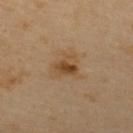Clinical impression:
Part of a total-body skin-imaging series; this lesion was reviewed on a skin check and was not flagged for biopsy.
Image and clinical context:
The recorded lesion diameter is about 3 mm. The subject is a male about 55 years old. This image is a 15 mm lesion crop taken from a total-body photograph. Located on the upper back. The lesion-visualizer software estimated a mean CIELAB color near L≈47 a*≈19 b*≈36, about 10 CIELAB-L* units darker than the surrounding skin, and a normalized border contrast of about 8.